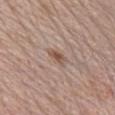  biopsy_status: not biopsied; imaged during a skin examination
  image:
    source: total-body photography crop
    field_of_view_mm: 15
  site: chest
  patient:
    sex: female
    age_approx: 65